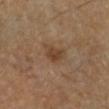Findings:
– follow-up — no biopsy performed (imaged during a skin exam)
– tile lighting — cross-polarized
– subject — male, aged approximately 60
– body site — the left lower leg
– diameter — ≈2.5 mm
– image — ~15 mm tile from a whole-body skin photo